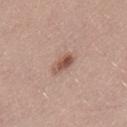Part of a total-body skin-imaging series; this lesion was reviewed on a skin check and was not flagged for biopsy. A male patient aged 48–52. A 15 mm close-up tile from a total-body photography series done for melanoma screening. This is a white-light tile. Longest diameter approximately 3 mm. The lesion is on the lower back. The total-body-photography lesion software estimated a footprint of about 4.5 mm², an eccentricity of roughly 0.85, and a symmetry-axis asymmetry near 0.2. The analysis additionally found a lesion color around L≈53 a*≈20 b*≈27 in CIELAB, roughly 12 lightness units darker than nearby skin, and a normalized border contrast of about 8.5. And it measured a classifier nevus-likeness of about 90/100 and a detector confidence of about 100 out of 100 that the crop contains a lesion.